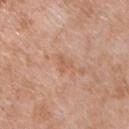Imaged during a routine full-body skin examination; the lesion was not biopsied and no histopathology is available. The subject is a male roughly 50 years of age. Longest diameter approximately 3 mm. Imaged with white-light lighting. Cropped from a total-body skin-imaging series; the visible field is about 15 mm. The lesion is located on the chest.